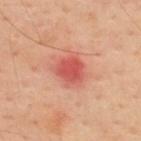Acquisition and patient details: About 3.5 mm across. A male subject, roughly 45 years of age. A 15 mm close-up extracted from a 3D total-body photography capture. The lesion is located on the upper back.